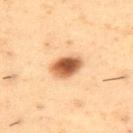Recorded during total-body skin imaging; not selected for excision or biopsy. The subject is a male approximately 55 years of age. The lesion is located on the back. A 15 mm close-up extracted from a 3D total-body photography capture.